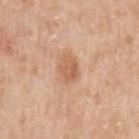The tile uses white-light illumination.
A male subject aged approximately 80.
A close-up tile cropped from a whole-body skin photograph, about 15 mm across.
An algorithmic analysis of the crop reported an area of roughly 6 mm² and a symmetry-axis asymmetry near 0.15.
Longest diameter approximately 3 mm.
From the left upper arm.